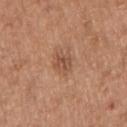- tile lighting: white-light
- imaging modality: 15 mm crop, total-body photography
- body site: the right upper arm
- diameter: about 2.5 mm
- subject: male, in their mid-70s
- image-analysis metrics: a footprint of about 4.5 mm² and a symmetry-axis asymmetry near 0.3; a border-irregularity rating of about 2.5/10, internal color variation of about 3 on a 0–10 scale, and a peripheral color-asymmetry measure near 1.5; a nevus-likeness score of about 50/100